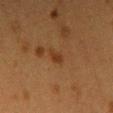size: ~2.5 mm (longest diameter) | body site: the back | subject: female, aged around 40 | lighting: cross-polarized | image-analysis metrics: an average lesion color of about L≈30 a*≈19 b*≈30 (CIELAB), a lesion–skin lightness drop of about 6, and a normalized border contrast of about 7; a border-irregularity index near 2.5/10, internal color variation of about 1 on a 0–10 scale, and peripheral color asymmetry of about 0.5 | imaging modality: ~15 mm tile from a whole-body skin photo.